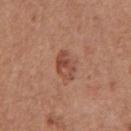Q: What is the imaging modality?
A: ~15 mm crop, total-body skin-cancer survey
Q: What are the patient's age and sex?
A: male, in their mid- to late 60s
Q: Lesion location?
A: the chest